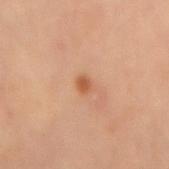biopsy_status: not biopsied; imaged during a skin examination
image:
  source: total-body photography crop
  field_of_view_mm: 15
site: lower back
lighting: cross-polarized
patient:
  sex: male
  age_approx: 55